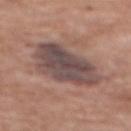Findings:
– workup — total-body-photography surveillance lesion; no biopsy
– subject — female, roughly 75 years of age
– body site — the upper back
– imaging modality — total-body-photography crop, ~15 mm field of view